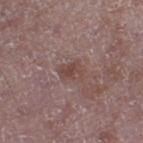Recorded during total-body skin imaging; not selected for excision or biopsy.
A close-up tile cropped from a whole-body skin photograph, about 15 mm across.
Imaged with white-light lighting.
Located on the left thigh.
The subject is a male in their 50s.